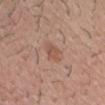workup: catalogued during a skin exam; not biopsied
lighting: white-light
image source: 15 mm crop, total-body photography
automated metrics: a lesion area of about 4 mm², an eccentricity of roughly 0.85, and a symmetry-axis asymmetry near 0.2; an average lesion color of about L≈53 a*≈21 b*≈29 (CIELAB) and a normalized border contrast of about 6
site: the head or neck
subject: male, aged 28 to 32
size: about 3 mm